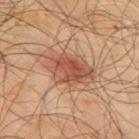This lesion was catalogued during total-body skin photography and was not selected for biopsy.
From the upper back.
A lesion tile, about 15 mm wide, cut from a 3D total-body photograph.
A male subject, in their mid- to late 60s.